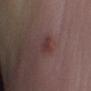This lesion was catalogued during total-body skin photography and was not selected for biopsy.
Automated tile analysis of the lesion measured a lesion area of about 3.5 mm², an outline eccentricity of about 0.3 (0 = round, 1 = elongated), and a symmetry-axis asymmetry near 0.3. And it measured an average lesion color of about L≈34 a*≈19 b*≈17 (CIELAB) and a lesion-to-skin contrast of about 6 (normalized; higher = more distinct). The software also gave internal color variation of about 2 on a 0–10 scale and peripheral color asymmetry of about 0.5.
A female patient in their 50s.
A close-up tile cropped from a whole-body skin photograph, about 15 mm across.
Longest diameter approximately 2 mm.
From the left lower leg.
Captured under white-light illumination.